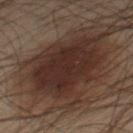Assessment: No biopsy was performed on this lesion — it was imaged during a full skin examination and was not determined to be concerning. Acquisition and patient details: Longest diameter approximately 9 mm. A close-up tile cropped from a whole-body skin photograph, about 15 mm across. From the right thigh. A male subject aged around 55.